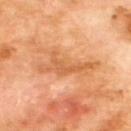Assessment:
Recorded during total-body skin imaging; not selected for excision or biopsy.
Context:
The patient is a male aged around 70. The lesion's longest dimension is about 7 mm. From the upper back. This image is a 15 mm lesion crop taken from a total-body photograph. Automated image analysis of the tile measured an area of roughly 15 mm².